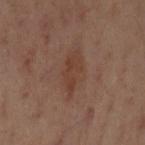{
  "biopsy_status": "not biopsied; imaged during a skin examination",
  "automated_metrics": {
    "cielab_L": 32,
    "cielab_a": 16,
    "cielab_b": 22,
    "vs_skin_darker_L": 5.0,
    "vs_skin_contrast_norm": 5.5,
    "border_irregularity_0_10": 2.5,
    "color_variation_0_10": 2.0,
    "nevus_likeness_0_100": 5,
    "lesion_detection_confidence_0_100": 100
  },
  "patient": {
    "sex": "female",
    "age_approx": 55
  },
  "lighting": "cross-polarized",
  "image": {
    "source": "total-body photography crop",
    "field_of_view_mm": 15
  },
  "site": "left upper arm"
}